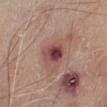| field | value |
|---|---|
| workup | catalogued during a skin exam; not biopsied |
| subject | male, approximately 65 years of age |
| lesion size | ~3.5 mm (longest diameter) |
| anatomic site | the left lower leg |
| acquisition | ~15 mm tile from a whole-body skin photo |
| tile lighting | white-light illumination |